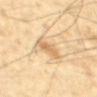biopsy status: no biopsy performed (imaged during a skin exam)
automated lesion analysis: a lesion area of about 6 mm² and an outline eccentricity of about 0.9 (0 = round, 1 = elongated); a within-lesion color-variation index near 2.5/10 and peripheral color asymmetry of about 1
tile lighting: cross-polarized
lesion diameter: about 4 mm
acquisition: ~15 mm crop, total-body skin-cancer survey
site: the back
patient: male, approximately 40 years of age